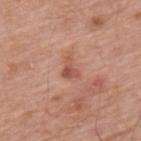follow-up — total-body-photography surveillance lesion; no biopsy | illumination — white-light | TBP lesion metrics — a lesion color around L≈53 a*≈26 b*≈30 in CIELAB, a lesion–skin lightness drop of about 10, and a normalized border contrast of about 7; a classifier nevus-likeness of about 0/100 and lesion-presence confidence of about 100/100 | subject — male, roughly 60 years of age | image source — 15 mm crop, total-body photography | size — about 2.5 mm | site — the upper back.